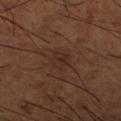Clinical impression:
The lesion was tiled from a total-body skin photograph and was not biopsied.
Clinical summary:
A male patient, aged 63 to 67. A roughly 15 mm field-of-view crop from a total-body skin photograph. Longest diameter approximately 3 mm. Imaged with cross-polarized lighting. Located on the right lower leg.